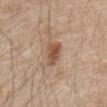Assessment: No biopsy was performed on this lesion — it was imaged during a full skin examination and was not determined to be concerning. Image and clinical context: Automated image analysis of the tile measured a lesion area of about 5.5 mm² and two-axis asymmetry of about 0.3. The analysis additionally found a nevus-likeness score of about 80/100. Cropped from a total-body skin-imaging series; the visible field is about 15 mm. A male subject aged approximately 80. Imaged with white-light lighting. Measured at roughly 3.5 mm in maximum diameter. Located on the abdomen.